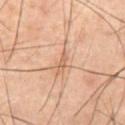Clinical impression: Part of a total-body skin-imaging series; this lesion was reviewed on a skin check and was not flagged for biopsy. Image and clinical context: The subject is a male aged approximately 60. This image is a 15 mm lesion crop taken from a total-body photograph. The lesion is on the abdomen. The lesion's longest dimension is about 2.5 mm. The tile uses cross-polarized illumination.